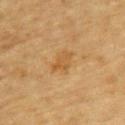{"biopsy_status": "not biopsied; imaged during a skin examination", "site": "upper back", "lighting": "cross-polarized", "image": {"source": "total-body photography crop", "field_of_view_mm": 15}, "automated_metrics": {"cielab_L": 48, "cielab_a": 17, "cielab_b": 38, "vs_skin_contrast_norm": 5.5, "border_irregularity_0_10": 4.0, "color_variation_0_10": 1.5, "peripheral_color_asymmetry": 0.5, "nevus_likeness_0_100": 0, "lesion_detection_confidence_0_100": 100}, "patient": {"sex": "male", "age_approx": "85+"}}